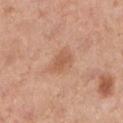A 15 mm close-up tile from a total-body photography series done for melanoma screening. Longest diameter approximately 3.5 mm. From the leg. This is a white-light tile. The patient is a female aged 38 to 42.